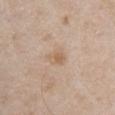Captured during whole-body skin photography for melanoma surveillance; the lesion was not biopsied.
Cropped from a total-body skin-imaging series; the visible field is about 15 mm.
On the right upper arm.
The tile uses white-light illumination.
About 2.5 mm across.
A male patient aged around 55.